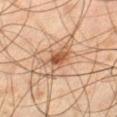Captured during whole-body skin photography for melanoma surveillance; the lesion was not biopsied. Located on the left thigh. This image is a 15 mm lesion crop taken from a total-body photograph. Imaged with cross-polarized lighting. The lesion-visualizer software estimated a mean CIELAB color near L≈47 a*≈17 b*≈28. It also reported a border-irregularity index near 6.5/10, internal color variation of about 5 on a 0–10 scale, and radial color variation of about 1. About 5.5 mm across. A male subject approximately 45 years of age.